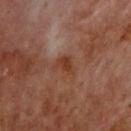Clinical impression: Part of a total-body skin-imaging series; this lesion was reviewed on a skin check and was not flagged for biopsy. Context: The lesion is on the back. Automated image analysis of the tile measured an area of roughly 4 mm², a shape eccentricity near 0.7, and two-axis asymmetry of about 0.45. The software also gave a classifier nevus-likeness of about 0/100 and a lesion-detection confidence of about 100/100. A 15 mm close-up tile from a total-body photography series done for melanoma screening. The tile uses cross-polarized illumination. A male subject, aged 68 to 72. Approximately 3 mm at its widest.